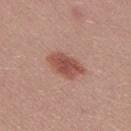workup: catalogued during a skin exam; not biopsied | subject: female, aged 23–27 | imaging modality: 15 mm crop, total-body photography | site: the leg | diameter: ~4.5 mm (longest diameter).